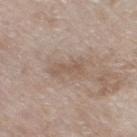<lesion>
<patient>
  <sex>female</sex>
  <age_approx>75</age_approx>
</patient>
<lighting>white-light</lighting>
<image>
  <source>total-body photography crop</source>
  <field_of_view_mm>15</field_of_view_mm>
</image>
<site>right thigh</site>
<lesion_size>
  <long_diameter_mm_approx>4.0</long_diameter_mm_approx>
</lesion_size>
</lesion>